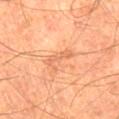Notes:
- follow-up: imaged on a skin check; not biopsied
- patient: male, roughly 65 years of age
- image source: ~15 mm tile from a whole-body skin photo
- lighting: cross-polarized
- automated metrics: a lesion area of about 5 mm², an outline eccentricity of about 0.85 (0 = round, 1 = elongated), and a shape-asymmetry score of about 0.5 (0 = symmetric); an average lesion color of about L≈60 a*≈25 b*≈36 (CIELAB), about 7 CIELAB-L* units darker than the surrounding skin, and a lesion-to-skin contrast of about 4.5 (normalized; higher = more distinct); a border-irregularity index near 7/10, internal color variation of about 0 on a 0–10 scale, and peripheral color asymmetry of about 0; an automated nevus-likeness rating near 0 out of 100 and lesion-presence confidence of about 100/100
- diameter: ≈3.5 mm
- site: the leg